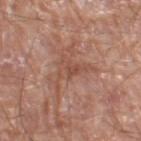Impression:
Part of a total-body skin-imaging series; this lesion was reviewed on a skin check and was not flagged for biopsy.
Acquisition and patient details:
A male patient roughly 80 years of age. From the left thigh. A close-up tile cropped from a whole-body skin photograph, about 15 mm across.